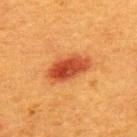<case>
<patient>
  <sex>female</sex>
  <age_approx>40</age_approx>
</patient>
<site>upper back</site>
<image>
  <source>total-body photography crop</source>
  <field_of_view_mm>15</field_of_view_mm>
</image>
</case>